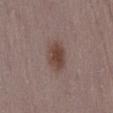| key | value |
|---|---|
| follow-up | catalogued during a skin exam; not biopsied |
| subject | female, about 50 years old |
| acquisition | ~15 mm tile from a whole-body skin photo |
| lighting | white-light |
| lesion size | ≈3.5 mm |
| location | the abdomen |